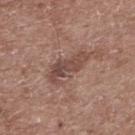<record>
<biopsy_status>not biopsied; imaged during a skin examination</biopsy_status>
<automated_metrics>
  <cielab_L>46</cielab_L>
  <cielab_a>19</cielab_a>
  <cielab_b>23</cielab_b>
  <vs_skin_darker_L>10.0</vs_skin_darker_L>
  <lesion_detection_confidence_0_100>100</lesion_detection_confidence_0_100>
</automated_metrics>
<lighting>white-light</lighting>
<patient>
  <sex>male</sex>
  <age_approx>60</age_approx>
</patient>
<lesion_size>
  <long_diameter_mm_approx>5.5</long_diameter_mm_approx>
</lesion_size>
<image>
  <source>total-body photography crop</source>
  <field_of_view_mm>15</field_of_view_mm>
</image>
<site>upper back</site>
</record>